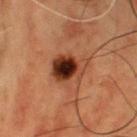<case>
  <biopsy_status>not biopsied; imaged during a skin examination</biopsy_status>
  <site>chest</site>
  <lighting>cross-polarized</lighting>
  <lesion_size>
    <long_diameter_mm_approx>4.0</long_diameter_mm_approx>
  </lesion_size>
  <image>
    <source>total-body photography crop</source>
    <field_of_view_mm>15</field_of_view_mm>
  </image>
  <patient>
    <sex>male</sex>
    <age_approx>50</age_approx>
  </patient>
</case>